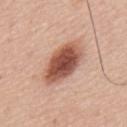follow-up: catalogued during a skin exam; not biopsied
acquisition: total-body-photography crop, ~15 mm field of view
lesion diameter: ~6 mm (longest diameter)
tile lighting: white-light illumination
location: the back
patient: male, aged around 65